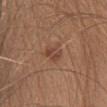<lesion>
<biopsy_status>not biopsied; imaged during a skin examination</biopsy_status>
<patient>
  <sex>female</sex>
  <age_approx>35</age_approx>
</patient>
<lesion_size>
  <long_diameter_mm_approx>2.5</long_diameter_mm_approx>
</lesion_size>
<lighting>white-light</lighting>
<site>head or neck</site>
<image>
  <source>total-body photography crop</source>
  <field_of_view_mm>15</field_of_view_mm>
</image>
</lesion>